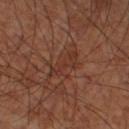The lesion was photographed on a routine skin check and not biopsied; there is no pathology result.
The tile uses cross-polarized illumination.
Automated tile analysis of the lesion measured a footprint of about 5.5 mm² and an eccentricity of roughly 0.95. It also reported a nevus-likeness score of about 0/100.
The recorded lesion diameter is about 4.5 mm.
A 15 mm close-up extracted from a 3D total-body photography capture.
Located on the left lower leg.
A male subject roughly 60 years of age.